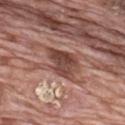The lesion was photographed on a routine skin check and not biopsied; there is no pathology result. The tile uses white-light illumination. The total-body-photography lesion software estimated an average lesion color of about L≈43 a*≈22 b*≈24 (CIELAB) and a lesion–skin lightness drop of about 14. The analysis additionally found a border-irregularity rating of about 4.5/10, a color-variation rating of about 4.5/10, and a peripheral color-asymmetry measure near 1.5. And it measured an automated nevus-likeness rating near 5 out of 100 and a lesion-detection confidence of about 85/100. The lesion is located on the mid back. A roughly 15 mm field-of-view crop from a total-body skin photograph. A male subject, roughly 70 years of age. About 4 mm across.